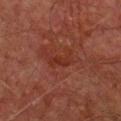Q: Is there a histopathology result?
A: imaged on a skin check; not biopsied
Q: Who is the patient?
A: male, in their mid-60s
Q: How large is the lesion?
A: ≈3.5 mm
Q: What is the imaging modality?
A: total-body-photography crop, ~15 mm field of view
Q: How was the tile lit?
A: cross-polarized illumination
Q: Automated lesion metrics?
A: a lesion area of about 5 mm², an eccentricity of roughly 0.85, and a symmetry-axis asymmetry near 0.4; a mean CIELAB color near L≈29 a*≈25 b*≈27 and a lesion-to-skin contrast of about 6 (normalized; higher = more distinct); a nevus-likeness score of about 0/100 and a detector confidence of about 100 out of 100 that the crop contains a lesion
Q: Lesion location?
A: the chest